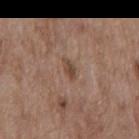Q: Was this lesion biopsied?
A: imaged on a skin check; not biopsied
Q: Patient demographics?
A: male, aged around 70
Q: What kind of image is this?
A: ~15 mm crop, total-body skin-cancer survey
Q: Where on the body is the lesion?
A: the back